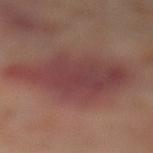Q: Was a biopsy performed?
A: no biopsy performed (imaged during a skin exam)
Q: What lighting was used for the tile?
A: cross-polarized
Q: What is the imaging modality?
A: total-body-photography crop, ~15 mm field of view
Q: What is the anatomic site?
A: the left lower leg
Q: Automated lesion metrics?
A: a shape eccentricity near 0.7; border irregularity of about 3.5 on a 0–10 scale, internal color variation of about 4 on a 0–10 scale, and peripheral color asymmetry of about 1.5
Q: Who is the patient?
A: female, aged around 55